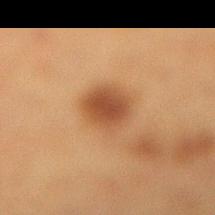The lesion was photographed on a routine skin check and not biopsied; there is no pathology result. The lesion's longest dimension is about 3.5 mm. A 15 mm crop from a total-body photograph taken for skin-cancer surveillance. A female patient, aged approximately 40. On the right lower leg. Imaged with cross-polarized lighting.